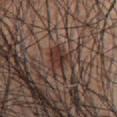Impression: No biopsy was performed on this lesion — it was imaged during a full skin examination and was not determined to be concerning. Acquisition and patient details: A 15 mm close-up extracted from a 3D total-body photography capture. The lesion is located on the abdomen. Captured under white-light illumination. The lesion's longest dimension is about 3.5 mm. The patient is a male in their mid- to late 50s. The lesion-visualizer software estimated a lesion area of about 7 mm² and two-axis asymmetry of about 0.4. And it measured a mean CIELAB color near L≈34 a*≈18 b*≈22 and a normalized lesion–skin contrast near 8. The software also gave a border-irregularity rating of about 4/10, a within-lesion color-variation index near 5.5/10, and radial color variation of about 1.5. The analysis additionally found an automated nevus-likeness rating near 95 out of 100 and a lesion-detection confidence of about 95/100.